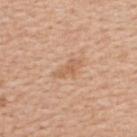Acquisition and patient details: A female subject in their 50s. This is a white-light tile. A 15 mm close-up extracted from a 3D total-body photography capture. From the back. The recorded lesion diameter is about 3.5 mm.